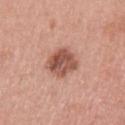Assessment: The lesion was photographed on a routine skin check and not biopsied; there is no pathology result. Context: Automated tile analysis of the lesion measured an outline eccentricity of about 0.55 (0 = round, 1 = elongated) and a shape-asymmetry score of about 0.2 (0 = symmetric). It also reported a border-irregularity rating of about 2/10 and radial color variation of about 2. A female subject aged 53 to 57. A lesion tile, about 15 mm wide, cut from a 3D total-body photograph. Imaged with white-light lighting. The lesion is on the arm.